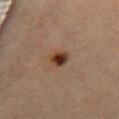Recorded during total-body skin imaging; not selected for excision or biopsy. The lesion is on the lower back. The patient is a female in their 40s. Cropped from a total-body skin-imaging series; the visible field is about 15 mm. The recorded lesion diameter is about 2.5 mm. This is a cross-polarized tile.